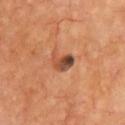image = ~15 mm tile from a whole-body skin photo | location = the chest.